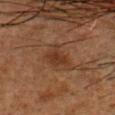notes = catalogued during a skin exam; not biopsied
patient = male, aged approximately 50
illumination = cross-polarized
lesion size = ≈3 mm
body site = the head or neck
image = ~15 mm tile from a whole-body skin photo